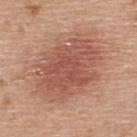Q: Was a biopsy performed?
A: catalogued during a skin exam; not biopsied